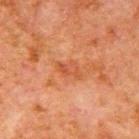{
  "image": {
    "source": "total-body photography crop",
    "field_of_view_mm": 15
  },
  "lesion_size": {
    "long_diameter_mm_approx": 3.0
  },
  "site": "right upper arm",
  "patient": {
    "sex": "male",
    "age_approx": 80
  }
}